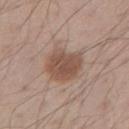Assessment:
This lesion was catalogued during total-body skin photography and was not selected for biopsy.
Clinical summary:
A male subject aged around 30. The lesion is on the right upper arm. A roughly 15 mm field-of-view crop from a total-body skin photograph. Measured at roughly 4.5 mm in maximum diameter. This is a white-light tile.